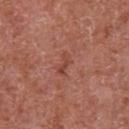Clinical impression:
This lesion was catalogued during total-body skin photography and was not selected for biopsy.
Image and clinical context:
Measured at roughly 3 mm in maximum diameter. Captured under white-light illumination. The total-body-photography lesion software estimated a mean CIELAB color near L≈45 a*≈27 b*≈29 and a normalized lesion–skin contrast near 5.5. The analysis additionally found a border-irregularity index near 4.5/10 and peripheral color asymmetry of about 0. And it measured a nevus-likeness score of about 0/100 and a lesion-detection confidence of about 100/100. A roughly 15 mm field-of-view crop from a total-body skin photograph. From the abdomen. A male patient, aged 63–67.